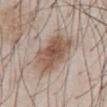The lesion was photographed on a routine skin check and not biopsied; there is no pathology result. A close-up tile cropped from a whole-body skin photograph, about 15 mm across. A male subject, in their mid-50s. The lesion is located on the abdomen.